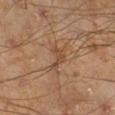Imaged during a routine full-body skin examination; the lesion was not biopsied and no histopathology is available.
A male patient, aged around 45.
This image is a 15 mm lesion crop taken from a total-body photograph.
Located on the right lower leg.